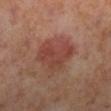The lesion was tiled from a total-body skin photograph and was not biopsied.
The lesion is located on the right lower leg.
The subject is a female aged 53–57.
The tile uses cross-polarized illumination.
A region of skin cropped from a whole-body photographic capture, roughly 15 mm wide.
Measured at roughly 5 mm in maximum diameter.